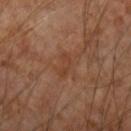Notes:
* notes — total-body-photography surveillance lesion; no biopsy
* illumination — cross-polarized
* patient — male, approximately 55 years of age
* body site — the left forearm
* image-analysis metrics — a symmetry-axis asymmetry near 0.4; a mean CIELAB color near L≈41 a*≈22 b*≈32 and a normalized lesion–skin contrast near 5.5; a border-irregularity index near 4/10 and a color-variation rating of about 0/10; a nevus-likeness score of about 0/100 and a detector confidence of about 100 out of 100 that the crop contains a lesion
* image source — ~15 mm crop, total-body skin-cancer survey
* lesion size — about 2.5 mm